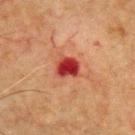biopsy status: no biopsy performed (imaged during a skin exam) | automated metrics: an area of roughly 5.5 mm², an outline eccentricity of about 0.55 (0 = round, 1 = elongated), and a symmetry-axis asymmetry near 0.2 | body site: the chest | illumination: cross-polarized | acquisition: 15 mm crop, total-body photography | subject: male, roughly 65 years of age | lesion size: ≈3 mm.